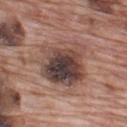| key | value |
|---|---|
| biopsy status | catalogued during a skin exam; not biopsied |
| anatomic site | the upper back |
| illumination | white-light illumination |
| patient | male, aged around 70 |
| acquisition | ~15 mm tile from a whole-body skin photo |